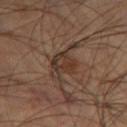Part of a total-body skin-imaging series; this lesion was reviewed on a skin check and was not flagged for biopsy.
A male patient about 55 years old.
On the left thigh.
Automated tile analysis of the lesion measured an area of roughly 6 mm², a shape eccentricity near 0.65, and two-axis asymmetry of about 0.25. And it measured an automated nevus-likeness rating near 0 out of 100 and lesion-presence confidence of about 95/100.
This is a cross-polarized tile.
The recorded lesion diameter is about 3 mm.
A lesion tile, about 15 mm wide, cut from a 3D total-body photograph.